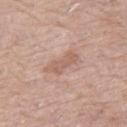{"biopsy_status": "not biopsied; imaged during a skin examination", "automated_metrics": {"area_mm2_approx": 5.0, "eccentricity": 0.9, "shape_asymmetry": 0.35, "cielab_L": 59, "cielab_a": 19, "cielab_b": 27, "vs_skin_darker_L": 8.0, "vs_skin_contrast_norm": 5.5, "nevus_likeness_0_100": 0, "lesion_detection_confidence_0_100": 100}, "image": {"source": "total-body photography crop", "field_of_view_mm": 15}, "site": "right thigh", "patient": {"sex": "female", "age_approx": 65}, "lesion_size": {"long_diameter_mm_approx": 4.0}}